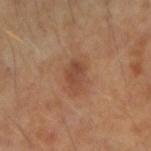Clinical impression: Recorded during total-body skin imaging; not selected for excision or biopsy. Acquisition and patient details: This is a cross-polarized tile. About 3.5 mm across. A 15 mm close-up tile from a total-body photography series done for melanoma screening. The lesion is located on the right forearm. The subject is a male approximately 45 years of age.